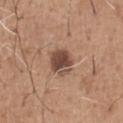| field | value |
|---|---|
| biopsy status | imaged on a skin check; not biopsied |
| diameter | ~3.5 mm (longest diameter) |
| patient | male, aged around 65 |
| TBP lesion metrics | a classifier nevus-likeness of about 65/100 and lesion-presence confidence of about 100/100 |
| acquisition | ~15 mm tile from a whole-body skin photo |
| anatomic site | the chest |